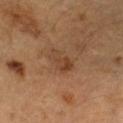Assessment: No biopsy was performed on this lesion — it was imaged during a full skin examination and was not determined to be concerning.